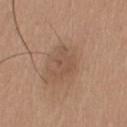No biopsy was performed on this lesion — it was imaged during a full skin examination and was not determined to be concerning.
On the left upper arm.
Automated image analysis of the tile measured a lesion area of about 7.5 mm², an eccentricity of roughly 0.6, and two-axis asymmetry of about 0.35.
A male patient about 40 years old.
Longest diameter approximately 3.5 mm.
Cropped from a total-body skin-imaging series; the visible field is about 15 mm.